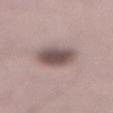Context:
A male subject, aged 68–72. A close-up tile cropped from a whole-body skin photograph, about 15 mm across. Captured under white-light illumination. Approximately 4.5 mm at its widest. The lesion is located on the lower back.